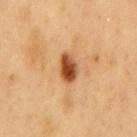<tbp_lesion>
  <biopsy_status>not biopsied; imaged during a skin examination</biopsy_status>
  <lesion_size>
    <long_diameter_mm_approx>3.5</long_diameter_mm_approx>
  </lesion_size>
  <site>chest</site>
  <image>
    <source>total-body photography crop</source>
    <field_of_view_mm>15</field_of_view_mm>
  </image>
  <lighting>cross-polarized</lighting>
  <patient>
    <sex>male</sex>
    <age_approx>60</age_approx>
  </patient>
</tbp_lesion>